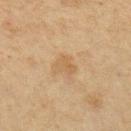* workup · total-body-photography surveillance lesion; no biopsy
* patient · female, aged 43 to 47
* image-analysis metrics · an eccentricity of roughly 0.6; an average lesion color of about L≈52 a*≈15 b*≈34 (CIELAB) and about 6 CIELAB-L* units darker than the surrounding skin
* anatomic site · the arm
* size · about 2.5 mm
* tile lighting · cross-polarized
* imaging modality · total-body-photography crop, ~15 mm field of view